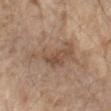Case summary:
* notes · no biopsy performed (imaged during a skin exam)
* acquisition · total-body-photography crop, ~15 mm field of view
* illumination · white-light illumination
* body site · the left forearm
* size · about 7.5 mm
* patient · female, aged around 85 or older